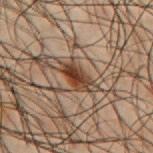The lesion was tiled from a total-body skin photograph and was not biopsied. The lesion is located on the mid back. Cropped from a total-body skin-imaging series; the visible field is about 15 mm. A male patient in their 50s. Approximately 3.5 mm at its widest. This is a cross-polarized tile.